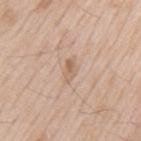Assessment:
Part of a total-body skin-imaging series; this lesion was reviewed on a skin check and was not flagged for biopsy.
Background:
On the mid back. A male subject, aged 63 to 67. Captured under white-light illumination. This image is a 15 mm lesion crop taken from a total-body photograph.